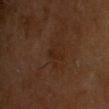Q: Was this lesion biopsied?
A: catalogued during a skin exam; not biopsied
Q: Where on the body is the lesion?
A: the head or neck
Q: What is the lesion's diameter?
A: about 3 mm
Q: Who is the patient?
A: male, roughly 60 years of age
Q: How was this image acquired?
A: 15 mm crop, total-body photography